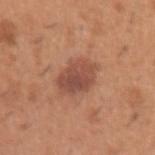follow-up — catalogued during a skin exam; not biopsied | image source — ~15 mm crop, total-body skin-cancer survey | diameter — ≈4.5 mm | body site — the left upper arm | subject — male, aged 38 to 42.